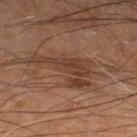The lesion was tiled from a total-body skin photograph and was not biopsied.
On the right thigh.
Measured at roughly 7.5 mm in maximum diameter.
Imaged with cross-polarized lighting.
A region of skin cropped from a whole-body photographic capture, roughly 15 mm wide.
A male patient aged 68–72.